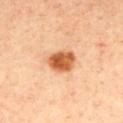Clinical impression: Captured during whole-body skin photography for melanoma surveillance; the lesion was not biopsied. Acquisition and patient details: The subject is a male roughly 40 years of age. Approximately 3.5 mm at its widest. The lesion is on the mid back. Automated tile analysis of the lesion measured an area of roughly 8 mm², a shape eccentricity near 0.7, and two-axis asymmetry of about 0.2. It also reported an average lesion color of about L≈61 a*≈30 b*≈43 (CIELAB) and about 18 CIELAB-L* units darker than the surrounding skin. A 15 mm close-up extracted from a 3D total-body photography capture. The tile uses cross-polarized illumination.